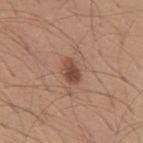  biopsy_status: not biopsied; imaged during a skin examination
  lesion_size:
    long_diameter_mm_approx: 3.0
  image:
    source: total-body photography crop
    field_of_view_mm: 15
  automated_metrics:
    nevus_likeness_0_100: 85
    lesion_detection_confidence_0_100: 100
  patient:
    sex: male
    age_approx: 30
  site: mid back